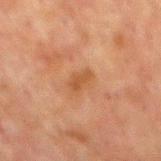site: the mid back
image source: ~15 mm tile from a whole-body skin photo
automated metrics: border irregularity of about 3 on a 0–10 scale, internal color variation of about 0 on a 0–10 scale, and peripheral color asymmetry of about 0; a nevus-likeness score of about 0/100 and a lesion-detection confidence of about 100/100
size: about 2.5 mm
patient: male, about 60 years old
illumination: cross-polarized illumination